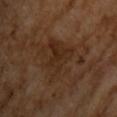  biopsy_status: not biopsied; imaged during a skin examination
  image:
    source: total-body photography crop
    field_of_view_mm: 15
  patient:
    sex: male
    age_approx: 65